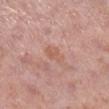Impression:
The lesion was tiled from a total-body skin photograph and was not biopsied.
Background:
A roughly 15 mm field-of-view crop from a total-body skin photograph. Automated image analysis of the tile measured a detector confidence of about 100 out of 100 that the crop contains a lesion. About 2.5 mm across. A female subject, aged approximately 50. From the leg.